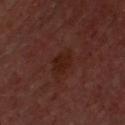This lesion was catalogued during total-body skin photography and was not selected for biopsy.
The lesion-visualizer software estimated a lesion area of about 4.5 mm² and a shape eccentricity near 0.7. The software also gave a border-irregularity index near 2/10 and a peripheral color-asymmetry measure near 0.5.
The subject is a male aged around 45.
On the chest.
The recorded lesion diameter is about 2.5 mm.
The tile uses cross-polarized illumination.
This image is a 15 mm lesion crop taken from a total-body photograph.